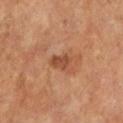This lesion was catalogued during total-body skin photography and was not selected for biopsy. The subject is a male in their mid-60s. Approximately 2.5 mm at its widest. Automated image analysis of the tile measured a footprint of about 3.5 mm². And it measured a mean CIELAB color near L≈45 a*≈24 b*≈33 and about 10 CIELAB-L* units darker than the surrounding skin. The tile uses cross-polarized illumination. The lesion is on the left lower leg. Cropped from a total-body skin-imaging series; the visible field is about 15 mm.